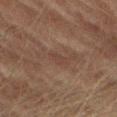Part of a total-body skin-imaging series; this lesion was reviewed on a skin check and was not flagged for biopsy. Cropped from a whole-body photographic skin survey; the tile spans about 15 mm. The lesion is located on the left thigh. The lesion's longest dimension is about 2.5 mm. Captured under cross-polarized illumination. A male patient, approximately 75 years of age.